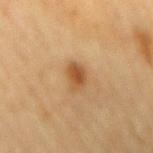Clinical impression:
Imaged during a routine full-body skin examination; the lesion was not biopsied and no histopathology is available.
Image and clinical context:
A female subject in their mid-50s. The lesion is located on the mid back. This is a cross-polarized tile. The recorded lesion diameter is about 3.5 mm. A close-up tile cropped from a whole-body skin photograph, about 15 mm across.